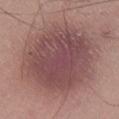{"biopsy_status": "not biopsied; imaged during a skin examination", "automated_metrics": {"nevus_likeness_0_100": 25, "lesion_detection_confidence_0_100": 100}, "lighting": "white-light", "image": {"source": "total-body photography crop", "field_of_view_mm": 15}, "site": "right lower leg", "lesion_size": {"long_diameter_mm_approx": 9.5}, "patient": {"sex": "male", "age_approx": 35}}